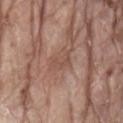Impression:
Imaged during a routine full-body skin examination; the lesion was not biopsied and no histopathology is available.
Acquisition and patient details:
The lesion is on the lower back. Cropped from a total-body skin-imaging series; the visible field is about 15 mm. Automated image analysis of the tile measured a lesion area of about 4 mm² and a shape eccentricity near 0.8. It also reported a lesion color around L≈50 a*≈19 b*≈27 in CIELAB, roughly 6 lightness units darker than nearby skin, and a normalized lesion–skin contrast near 4.5. And it measured a nevus-likeness score of about 0/100 and a lesion-detection confidence of about 95/100. A male subject, roughly 80 years of age.